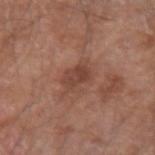This lesion was catalogued during total-body skin photography and was not selected for biopsy.
From the right upper arm.
A close-up tile cropped from a whole-body skin photograph, about 15 mm across.
A male patient about 65 years old.